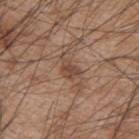Clinical impression:
Recorded during total-body skin imaging; not selected for excision or biopsy.
Clinical summary:
Located on the upper back. This is a white-light tile. Longest diameter approximately 3.5 mm. A close-up tile cropped from a whole-body skin photograph, about 15 mm across. A male subject about 45 years old.